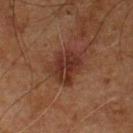biopsy_status: not biopsied; imaged during a skin examination
lesion_size:
  long_diameter_mm_approx: 3.5
lighting: cross-polarized
automated_metrics:
  cielab_L: 29
  cielab_a: 21
  cielab_b: 24
  vs_skin_darker_L: 9.0
  vs_skin_contrast_norm: 9.0
  border_irregularity_0_10: 3.0
  color_variation_0_10: 4.0
  peripheral_color_asymmetry: 1.5
  nevus_likeness_0_100: 55
site: chest
patient:
  sex: male
  age_approx: 55
image:
  source: total-body photography crop
  field_of_view_mm: 15